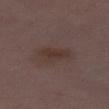Impression: No biopsy was performed on this lesion — it was imaged during a full skin examination and was not determined to be concerning. Context: A 15 mm close-up extracted from a 3D total-body photography capture. About 4 mm across. The lesion-visualizer software estimated a footprint of about 7 mm², a shape eccentricity near 0.85, and a symmetry-axis asymmetry near 0.3. And it measured a mean CIELAB color near L≈34 a*≈15 b*≈19 and roughly 6 lightness units darker than nearby skin. It also reported a border-irregularity index near 3/10, a color-variation rating of about 2.5/10, and a peripheral color-asymmetry measure near 0.5. The software also gave a classifier nevus-likeness of about 10/100 and lesion-presence confidence of about 100/100. From the right thigh. Captured under white-light illumination. A female patient aged 28–32.